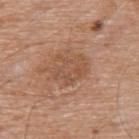| feature | finding |
|---|---|
| notes | total-body-photography surveillance lesion; no biopsy |
| location | the upper back |
| patient | male, aged approximately 80 |
| acquisition | total-body-photography crop, ~15 mm field of view |
| size | ≈4.5 mm |
| tile lighting | white-light illumination |